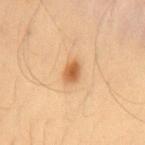Impression:
This lesion was catalogued during total-body skin photography and was not selected for biopsy.
Clinical summary:
The recorded lesion diameter is about 3 mm. A 15 mm close-up tile from a total-body photography series done for melanoma screening. A male subject about 55 years old. The tile uses cross-polarized illumination. The lesion is on the mid back. Automated image analysis of the tile measured a lesion color around L≈59 a*≈23 b*≈41 in CIELAB, about 13 CIELAB-L* units darker than the surrounding skin, and a lesion-to-skin contrast of about 8.5 (normalized; higher = more distinct). The software also gave a border-irregularity rating of about 2/10, a within-lesion color-variation index near 3.5/10, and peripheral color asymmetry of about 1. And it measured an automated nevus-likeness rating near 100 out of 100 and a detector confidence of about 100 out of 100 that the crop contains a lesion.